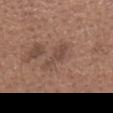Findings:
– notes: imaged on a skin check; not biopsied
– body site: the head or neck
– patient: male, in their mid-60s
– lesion size: ~4.5 mm (longest diameter)
– tile lighting: white-light illumination
– image: ~15 mm crop, total-body skin-cancer survey